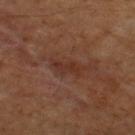Captured during whole-body skin photography for melanoma surveillance; the lesion was not biopsied.
Approximately 4 mm at its widest.
This is a cross-polarized tile.
This image is a 15 mm lesion crop taken from a total-body photograph.
A male patient, approximately 60 years of age.
The lesion-visualizer software estimated a footprint of about 5 mm² and an eccentricity of roughly 0.9. The software also gave a mean CIELAB color near L≈29 a*≈20 b*≈24, about 6 CIELAB-L* units darker than the surrounding skin, and a normalized lesion–skin contrast near 6. The software also gave peripheral color asymmetry of about 1. The analysis additionally found an automated nevus-likeness rating near 0 out of 100.
On the upper back.